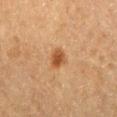Notes:
* notes: total-body-photography surveillance lesion; no biopsy
* subject: male, aged approximately 75
* tile lighting: cross-polarized
* site: the leg
* TBP lesion metrics: a border-irregularity index near 2/10 and radial color variation of about 0.5; a classifier nevus-likeness of about 95/100 and a lesion-detection confidence of about 100/100
* imaging modality: 15 mm crop, total-body photography